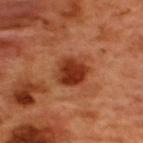{"biopsy_status": "not biopsied; imaged during a skin examination", "image": {"source": "total-body photography crop", "field_of_view_mm": 15}, "patient": {"sex": "male", "age_approx": 50}, "site": "back", "lesion_size": {"long_diameter_mm_approx": 4.0}, "lighting": "cross-polarized"}